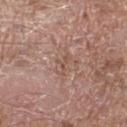image:
  source: total-body photography crop
  field_of_view_mm: 15
lesion_size:
  long_diameter_mm_approx: 3.0
site: left thigh
patient:
  sex: male
  age_approx: 65